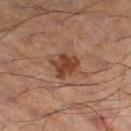Findings:
– follow-up — total-body-photography surveillance lesion; no biopsy
– image — ~15 mm tile from a whole-body skin photo
– body site — the left lower leg
– subject — male, in their mid- to late 50s